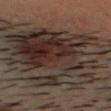subject: male, in their 30s | site: the head or neck | imaging modality: ~15 mm tile from a whole-body skin photo.